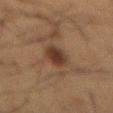The lesion was tiled from a total-body skin photograph and was not biopsied. The lesion is on the back. A male subject, about 60 years old. An algorithmic analysis of the crop reported a lesion–skin lightness drop of about 9 and a normalized border contrast of about 9. Cropped from a whole-body photographic skin survey; the tile spans about 15 mm. The tile uses cross-polarized illumination.